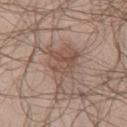| feature | finding |
|---|---|
| imaging modality | ~15 mm crop, total-body skin-cancer survey |
| subject | male, in their 40s |
| TBP lesion metrics | a footprint of about 15 mm², a shape eccentricity near 0.6, and a symmetry-axis asymmetry near 0.45 |
| site | the chest |
| illumination | white-light |
| size | ≈6 mm |